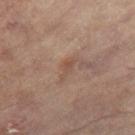Notes:
– notes · catalogued during a skin exam; not biopsied
– subject · female, about 80 years old
– tile lighting · cross-polarized
– image · 15 mm crop, total-body photography
– diameter · ~3 mm (longest diameter)
– body site · the left leg